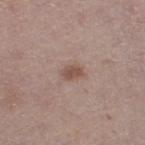Assessment: Part of a total-body skin-imaging series; this lesion was reviewed on a skin check and was not flagged for biopsy. Image and clinical context: Measured at roughly 2.5 mm in maximum diameter. This is a white-light tile. Automated image analysis of the tile measured border irregularity of about 1.5 on a 0–10 scale, internal color variation of about 1.5 on a 0–10 scale, and a peripheral color-asymmetry measure near 0.5. A 15 mm close-up tile from a total-body photography series done for melanoma screening. A female patient, in their 50s. On the left thigh.